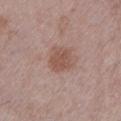biopsy status: total-body-photography surveillance lesion; no biopsy | image source: ~15 mm crop, total-body skin-cancer survey | subject: female, aged approximately 60 | lesion size: ≈3 mm | body site: the left lower leg.